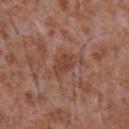  biopsy_status: not biopsied; imaged during a skin examination
  lighting: white-light
  lesion_size:
    long_diameter_mm_approx: 3.0
  image:
    source: total-body photography crop
    field_of_view_mm: 15
  patient:
    sex: male
    age_approx: 45
  site: chest